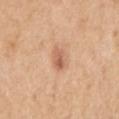workup — no biopsy performed (imaged during a skin exam)
illumination — white-light
acquisition — ~15 mm tile from a whole-body skin photo
location — the right upper arm
subject — female, aged 48 to 52
diameter — ~2.5 mm (longest diameter)